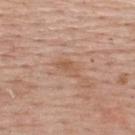Impression: The lesion was tiled from a total-body skin photograph and was not biopsied. Clinical summary: Cropped from a total-body skin-imaging series; the visible field is about 15 mm. A female patient aged 63–67. Captured under white-light illumination. The lesion is on the upper back.